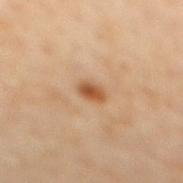Part of a total-body skin-imaging series; this lesion was reviewed on a skin check and was not flagged for biopsy. Imaged with cross-polarized lighting. From the back. The lesion's longest dimension is about 2.5 mm. A male subject in their mid- to late 60s. This image is a 15 mm lesion crop taken from a total-body photograph.